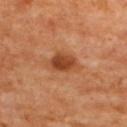This lesion was catalogued during total-body skin photography and was not selected for biopsy.
A female patient, aged 63 to 67.
Cropped from a total-body skin-imaging series; the visible field is about 15 mm.
The lesion's longest dimension is about 4 mm.
The total-body-photography lesion software estimated a footprint of about 8 mm². The analysis additionally found an average lesion color of about L≈45 a*≈26 b*≈36 (CIELAB) and roughly 11 lightness units darker than nearby skin. It also reported a within-lesion color-variation index near 4.5/10. And it measured lesion-presence confidence of about 100/100.
On the upper back.
Imaged with cross-polarized lighting.